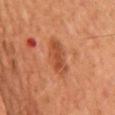Clinical impression:
Imaged during a routine full-body skin examination; the lesion was not biopsied and no histopathology is available.